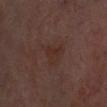Q: What kind of image is this?
A: ~15 mm tile from a whole-body skin photo
Q: Where on the body is the lesion?
A: the head or neck
Q: How large is the lesion?
A: about 3.5 mm
Q: Who is the patient?
A: roughly 65 years of age
Q: Illumination type?
A: cross-polarized